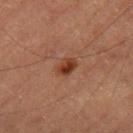{
  "biopsy_status": "not biopsied; imaged during a skin examination",
  "automated_metrics": {
    "area_mm2_approx": 4.0,
    "shape_asymmetry": 0.2,
    "lesion_detection_confidence_0_100": 100
  },
  "lighting": "cross-polarized",
  "patient": {
    "sex": "male",
    "age_approx": "85+"
  },
  "site": "right thigh",
  "image": {
    "source": "total-body photography crop",
    "field_of_view_mm": 15
  },
  "lesion_size": {
    "long_diameter_mm_approx": 2.5
  }
}